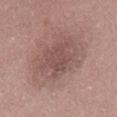biopsy status = catalogued during a skin exam; not biopsied
image source = 15 mm crop, total-body photography
anatomic site = the lower back
diameter = ≈4 mm
patient = male, about 30 years old
illumination = white-light